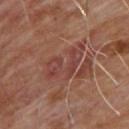Part of a total-body skin-imaging series; this lesion was reviewed on a skin check and was not flagged for biopsy. A male patient, aged approximately 65. Cropped from a whole-body photographic skin survey; the tile spans about 15 mm. Located on the upper back.